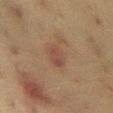From the abdomen.
Longest diameter approximately 3 mm.
A 15 mm close-up extracted from a 3D total-body photography capture.
This is a cross-polarized tile.
The total-body-photography lesion software estimated a border-irregularity index near 1.5/10 and internal color variation of about 2.5 on a 0–10 scale. The analysis additionally found a nevus-likeness score of about 20/100 and lesion-presence confidence of about 100/100.
A female patient about 40 years old.